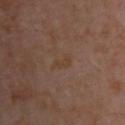– workup — imaged on a skin check; not biopsied
– illumination — cross-polarized illumination
– size — ~3 mm (longest diameter)
– image — 15 mm crop, total-body photography
– automated lesion analysis — an area of roughly 3 mm², an eccentricity of roughly 0.9, and two-axis asymmetry of about 0.35; a lesion color around L≈38 a*≈16 b*≈27 in CIELAB, a lesion–skin lightness drop of about 4, and a lesion-to-skin contrast of about 5.5 (normalized; higher = more distinct); a border-irregularity index near 4.5/10 and radial color variation of about 0; an automated nevus-likeness rating near 0 out of 100 and a detector confidence of about 100 out of 100 that the crop contains a lesion
– location — the back
– subject — aged approximately 55